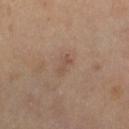follow-up: imaged on a skin check; not biopsied
illumination: cross-polarized
body site: the left thigh
diameter: about 2.5 mm
subject: female, aged 53 to 57
acquisition: ~15 mm tile from a whole-body skin photo
image-analysis metrics: a classifier nevus-likeness of about 0/100